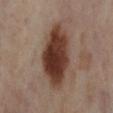Clinical impression:
Part of a total-body skin-imaging series; this lesion was reviewed on a skin check and was not flagged for biopsy.
Image and clinical context:
This is a cross-polarized tile. Located on the left lower leg. A region of skin cropped from a whole-body photographic capture, roughly 15 mm wide. A female subject, roughly 55 years of age. Automated image analysis of the tile measured a lesion area of about 27 mm², an eccentricity of roughly 0.85, and a symmetry-axis asymmetry near 0.15. It also reported lesion-presence confidence of about 100/100.